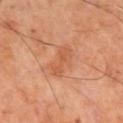Assessment: The lesion was photographed on a routine skin check and not biopsied; there is no pathology result. Context: A lesion tile, about 15 mm wide, cut from a 3D total-body photograph. A male patient aged approximately 70. Imaged with cross-polarized lighting. From the right lower leg. Longest diameter approximately 4 mm.